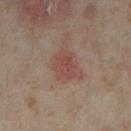Clinical impression: The lesion was photographed on a routine skin check and not biopsied; there is no pathology result. Image and clinical context: The recorded lesion diameter is about 3.5 mm. The patient is a female in their mid-30s. On the left lower leg. A region of skin cropped from a whole-body photographic capture, roughly 15 mm wide. This is a cross-polarized tile.